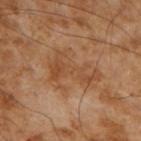Imaged during a routine full-body skin examination; the lesion was not biopsied and no histopathology is available. Captured under cross-polarized illumination. Located on the right upper arm. The patient is a male in their mid-50s. The lesion's longest dimension is about 6 mm. A 15 mm close-up extracted from a 3D total-body photography capture.